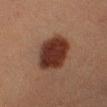<case>
<biopsy_status>not biopsied; imaged during a skin examination</biopsy_status>
<patient>
  <sex>male</sex>
  <age_approx>45</age_approx>
</patient>
<image>
  <source>total-body photography crop</source>
  <field_of_view_mm>15</field_of_view_mm>
</image>
<site>leg</site>
</case>